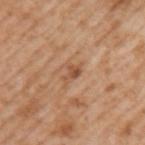Clinical impression:
Part of a total-body skin-imaging series; this lesion was reviewed on a skin check and was not flagged for biopsy.
Clinical summary:
Automated tile analysis of the lesion measured an average lesion color of about L≈53 a*≈22 b*≈34 (CIELAB), roughly 9 lightness units darker than nearby skin, and a normalized lesion–skin contrast near 6.5. The analysis additionally found a border-irregularity index near 5/10, internal color variation of about 1.5 on a 0–10 scale, and peripheral color asymmetry of about 0.5. Located on the left upper arm. Imaged with white-light lighting. A male subject roughly 65 years of age. A 15 mm close-up tile from a total-body photography series done for melanoma screening. Approximately 3 mm at its widest.